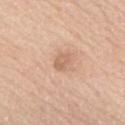Assessment: Part of a total-body skin-imaging series; this lesion was reviewed on a skin check and was not flagged for biopsy. Context: On the upper back. Cropped from a whole-body photographic skin survey; the tile spans about 15 mm. A male patient, approximately 75 years of age. The lesion-visualizer software estimated a border-irregularity index near 2.5/10. The analysis additionally found a nevus-likeness score of about 0/100 and a detector confidence of about 100 out of 100 that the crop contains a lesion. About 2.5 mm across. Captured under white-light illumination.